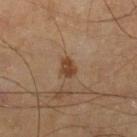Assessment: The lesion was tiled from a total-body skin photograph and was not biopsied. Acquisition and patient details: A male patient aged around 60. Measured at roughly 2.5 mm in maximum diameter. Located on the left lower leg. An algorithmic analysis of the crop reported a mean CIELAB color near L≈37 a*≈17 b*≈29, about 9 CIELAB-L* units darker than the surrounding skin, and a normalized lesion–skin contrast near 9. And it measured a border-irregularity rating of about 2.5/10, internal color variation of about 1.5 on a 0–10 scale, and a peripheral color-asymmetry measure near 0.5. This image is a 15 mm lesion crop taken from a total-body photograph.